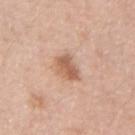Clinical impression:
This lesion was catalogued during total-body skin photography and was not selected for biopsy.
Clinical summary:
A 15 mm close-up extracted from a 3D total-body photography capture. The subject is a female aged around 40. From the left forearm. This is a white-light tile.